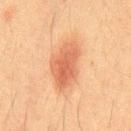Findings:
• biopsy status · no biopsy performed (imaged during a skin exam)
• lesion size · about 6 mm
• image · total-body-photography crop, ~15 mm field of view
• patient · male, in their mid-30s
• location · the chest